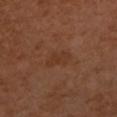Q: Was a biopsy performed?
A: total-body-photography surveillance lesion; no biopsy
Q: What is the anatomic site?
A: the left forearm
Q: Lesion size?
A: ≈3.5 mm
Q: What are the patient's age and sex?
A: female, roughly 55 years of age
Q: What lighting was used for the tile?
A: cross-polarized illumination
Q: How was this image acquired?
A: 15 mm crop, total-body photography